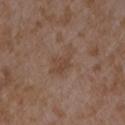Q: Was a biopsy performed?
A: imaged on a skin check; not biopsied
Q: Automated lesion metrics?
A: an area of roughly 7 mm², an eccentricity of roughly 0.8, and a shape-asymmetry score of about 0.4 (0 = symmetric); a mean CIELAB color near L≈44 a*≈17 b*≈26; a nevus-likeness score of about 0/100 and a detector confidence of about 100 out of 100 that the crop contains a lesion
Q: What kind of image is this?
A: ~15 mm tile from a whole-body skin photo
Q: How large is the lesion?
A: about 4 mm
Q: Lesion location?
A: the arm
Q: What lighting was used for the tile?
A: white-light
Q: Who is the patient?
A: female, approximately 35 years of age